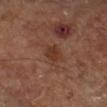Recorded during total-body skin imaging; not selected for excision or biopsy. Located on the right lower leg. A subject aged 63 to 67. A region of skin cropped from a whole-body photographic capture, roughly 15 mm wide.